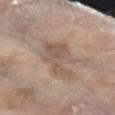{
  "lighting": "white-light",
  "site": "arm",
  "lesion_size": {
    "long_diameter_mm_approx": 6.0
  },
  "image": {
    "source": "total-body photography crop",
    "field_of_view_mm": 15
  },
  "patient": {
    "sex": "female",
    "age_approx": 70
  }
}